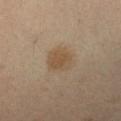Captured during whole-body skin photography for melanoma surveillance; the lesion was not biopsied.
The lesion is on the left forearm.
This image is a 15 mm lesion crop taken from a total-body photograph.
Measured at roughly 3.5 mm in maximum diameter.
A male subject approximately 55 years of age.